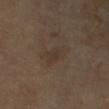This lesion was catalogued during total-body skin photography and was not selected for biopsy. The lesion is on the right upper arm. Imaged with cross-polarized lighting. The subject is a male roughly 70 years of age. The lesion's longest dimension is about 4 mm. The lesion-visualizer software estimated an average lesion color of about L≈33 a*≈12 b*≈21 (CIELAB), roughly 4 lightness units darker than nearby skin, and a lesion-to-skin contrast of about 4.5 (normalized; higher = more distinct). And it measured a within-lesion color-variation index near 3/10 and radial color variation of about 1. The analysis additionally found a classifier nevus-likeness of about 0/100 and a lesion-detection confidence of about 100/100. Cropped from a whole-body photographic skin survey; the tile spans about 15 mm.